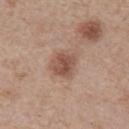Impression: Captured during whole-body skin photography for melanoma surveillance; the lesion was not biopsied. Acquisition and patient details: On the chest. A close-up tile cropped from a whole-body skin photograph, about 15 mm across. The tile uses white-light illumination. The lesion-visualizer software estimated a footprint of about 8.5 mm², an outline eccentricity of about 0.4 (0 = round, 1 = elongated), and a symmetry-axis asymmetry near 0.2. The software also gave a lesion color around L≈52 a*≈20 b*≈27 in CIELAB, about 11 CIELAB-L* units darker than the surrounding skin, and a normalized border contrast of about 7.5. It also reported a border-irregularity rating of about 2/10 and a peripheral color-asymmetry measure near 1.5. A male patient, aged 63 to 67. The lesion's longest dimension is about 3.5 mm.